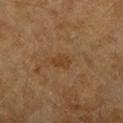No biopsy was performed on this lesion — it was imaged during a full skin examination and was not determined to be concerning. Captured under cross-polarized illumination. The patient is a female aged 58 to 62. Automated image analysis of the tile measured a nevus-likeness score of about 5/100. Longest diameter approximately 2.5 mm. A 15 mm close-up extracted from a 3D total-body photography capture. The lesion is located on the arm.